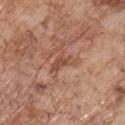| field | value |
|---|---|
| follow-up | imaged on a skin check; not biopsied |
| subject | male, about 70 years old |
| image source | ~15 mm crop, total-body skin-cancer survey |
| anatomic site | the chest |
| tile lighting | white-light |